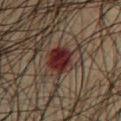Recorded during total-body skin imaging; not selected for excision or biopsy. Measured at roughly 4 mm in maximum diameter. A lesion tile, about 15 mm wide, cut from a 3D total-body photograph. The total-body-photography lesion software estimated a lesion area of about 9.5 mm². It also reported a border-irregularity index near 2.5/10 and internal color variation of about 4.5 on a 0–10 scale. Imaged with cross-polarized lighting. The lesion is on the chest. A male subject, aged around 65.